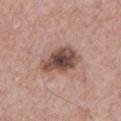follow-up — total-body-photography surveillance lesion; no biopsy | acquisition — 15 mm crop, total-body photography | subject — male, in their mid- to late 50s | illumination — white-light.